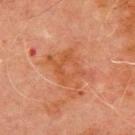notes — no biopsy performed (imaged during a skin exam) | illumination — cross-polarized illumination | subject — male, aged 68–72 | location — the chest | acquisition — ~15 mm crop, total-body skin-cancer survey.